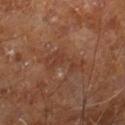Assessment:
Captured during whole-body skin photography for melanoma surveillance; the lesion was not biopsied.
Background:
Captured under cross-polarized illumination. From the right leg. A close-up tile cropped from a whole-body skin photograph, about 15 mm across. A male subject, aged around 60. The total-body-photography lesion software estimated a lesion area of about 7 mm² and a symmetry-axis asymmetry near 0.55.